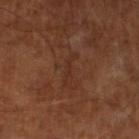Impression: Captured during whole-body skin photography for melanoma surveillance; the lesion was not biopsied. Background: The subject is a male aged around 65. The lesion is located on the left lower leg. Approximately 4 mm at its widest. This is a cross-polarized tile. A lesion tile, about 15 mm wide, cut from a 3D total-body photograph.